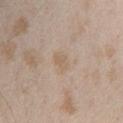The lesion was photographed on a routine skin check and not biopsied; there is no pathology result.
A female subject approximately 25 years of age.
A region of skin cropped from a whole-body photographic capture, roughly 15 mm wide.
Located on the upper back.
This is a white-light tile.
Measured at roughly 2.5 mm in maximum diameter.
Automated tile analysis of the lesion measured a border-irregularity index near 1.5/10, internal color variation of about 1.5 on a 0–10 scale, and peripheral color asymmetry of about 0.5. The analysis additionally found an automated nevus-likeness rating near 0 out of 100 and a detector confidence of about 100 out of 100 that the crop contains a lesion.